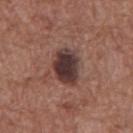  biopsy_status: not biopsied; imaged during a skin examination
  patient:
    sex: male
    age_approx: 75
  lighting: white-light
  automated_metrics:
    area_mm2_approx: 11.0
    eccentricity: 0.6
    shape_asymmetry: 0.2
    cielab_L: 35
    cielab_a: 18
    cielab_b: 18
    vs_skin_darker_L: 15.0
    vs_skin_contrast_norm: 13.5
  image:
    source: total-body photography crop
    field_of_view_mm: 15
  site: abdomen